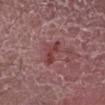- follow-up: imaged on a skin check; not biopsied
- illumination: white-light
- location: the left lower leg
- lesion diameter: ≈3.5 mm
- image source: ~15 mm crop, total-body skin-cancer survey
- patient: male, aged around 45